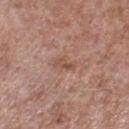follow-up=no biopsy performed (imaged during a skin exam); illumination=white-light; size=~2.5 mm (longest diameter); subject=male, in their mid- to late 50s; imaging modality=~15 mm tile from a whole-body skin photo; location=the right lower leg.